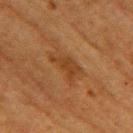Q: Is there a histopathology result?
A: imaged on a skin check; not biopsied
Q: What kind of image is this?
A: total-body-photography crop, ~15 mm field of view
Q: What are the patient's age and sex?
A: female, approximately 55 years of age
Q: Lesion size?
A: ~4.5 mm (longest diameter)
Q: How was the tile lit?
A: cross-polarized illumination
Q: Lesion location?
A: the arm